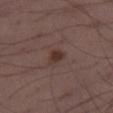No biopsy was performed on this lesion — it was imaged during a full skin examination and was not determined to be concerning. The tile uses white-light illumination. Located on the right thigh. A region of skin cropped from a whole-body photographic capture, roughly 15 mm wide. Longest diameter approximately 2.5 mm. Automated tile analysis of the lesion measured a lesion area of about 4 mm², a shape eccentricity near 0.6, and two-axis asymmetry of about 0.3. The analysis additionally found a classifier nevus-likeness of about 95/100 and a detector confidence of about 100 out of 100 that the crop contains a lesion. A male patient roughly 40 years of age.